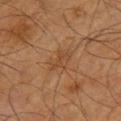Q: Is there a histopathology result?
A: imaged on a skin check; not biopsied
Q: How was the tile lit?
A: cross-polarized
Q: What is the imaging modality?
A: total-body-photography crop, ~15 mm field of view
Q: Where on the body is the lesion?
A: the left upper arm
Q: Who is the patient?
A: male, about 65 years old